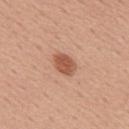Background:
This is a white-light tile. Located on the back. Automated tile analysis of the lesion measured roughly 12 lightness units darker than nearby skin and a lesion-to-skin contrast of about 8.5 (normalized; higher = more distinct). And it measured a border-irregularity index near 2/10, a within-lesion color-variation index near 2.5/10, and a peripheral color-asymmetry measure near 1. Longest diameter approximately 3 mm. A male subject aged 53 to 57. A roughly 15 mm field-of-view crop from a total-body skin photograph.